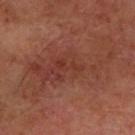Imaged during a routine full-body skin examination; the lesion was not biopsied and no histopathology is available.
Located on the left forearm.
Captured under cross-polarized illumination.
The patient is a male aged 58 to 62.
About 11.5 mm across.
A roughly 15 mm field-of-view crop from a total-body skin photograph.